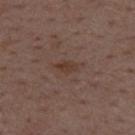No biopsy was performed on this lesion — it was imaged during a full skin examination and was not determined to be concerning.
The lesion is on the back.
A close-up tile cropped from a whole-body skin photograph, about 15 mm across.
A male subject aged around 50.